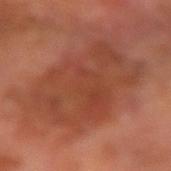{
  "biopsy_status": "not biopsied; imaged during a skin examination",
  "image": {
    "source": "total-body photography crop",
    "field_of_view_mm": 15
  },
  "site": "arm",
  "lesion_size": {
    "long_diameter_mm_approx": 9.0
  },
  "patient": {
    "sex": "male",
    "age_approx": 70
  }
}